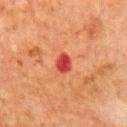No biopsy was performed on this lesion — it was imaged during a full skin examination and was not determined to be concerning. Measured at roughly 2.5 mm in maximum diameter. A lesion tile, about 15 mm wide, cut from a 3D total-body photograph. A male patient approximately 80 years of age. This is a cross-polarized tile. The lesion is located on the chest.